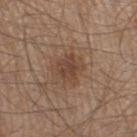Case summary:
- follow-up · imaged on a skin check; not biopsied
- anatomic site · the left thigh
- tile lighting · white-light illumination
- diameter · ~3 mm (longest diameter)
- imaging modality · total-body-photography crop, ~15 mm field of view
- patient · male, about 60 years old
- automated metrics · a lesion area of about 6 mm², a shape eccentricity near 0.35, and a shape-asymmetry score of about 0.3 (0 = symmetric); lesion-presence confidence of about 100/100